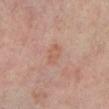Captured during whole-body skin photography for melanoma surveillance; the lesion was not biopsied. A male subject, approximately 65 years of age. The lesion is located on the leg. A lesion tile, about 15 mm wide, cut from a 3D total-body photograph.